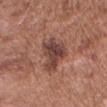workup — no biopsy performed (imaged during a skin exam)
size — ≈5.5 mm
subject — male, aged approximately 50
acquisition — 15 mm crop, total-body photography
automated lesion analysis — a mean CIELAB color near L≈43 a*≈21 b*≈25 and a lesion-to-skin contrast of about 9 (normalized; higher = more distinct); a border-irregularity rating of about 4.5/10, internal color variation of about 4.5 on a 0–10 scale, and radial color variation of about 1.5
tile lighting — white-light illumination
anatomic site — the head or neck